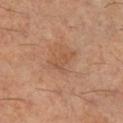{
  "biopsy_status": "not biopsied; imaged during a skin examination",
  "patient": {
    "sex": "male",
    "age_approx": 60
  },
  "automated_metrics": {
    "nevus_likeness_0_100": 0,
    "lesion_detection_confidence_0_100": 100
  },
  "image": {
    "source": "total-body photography crop",
    "field_of_view_mm": 15
  },
  "lighting": "cross-polarized",
  "lesion_size": {
    "long_diameter_mm_approx": 3.5
  },
  "site": "right lower leg"
}